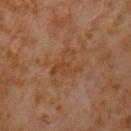Q: Is there a histopathology result?
A: imaged on a skin check; not biopsied
Q: What kind of image is this?
A: 15 mm crop, total-body photography
Q: How large is the lesion?
A: about 4 mm
Q: Lesion location?
A: the chest
Q: Patient demographics?
A: male, aged approximately 60